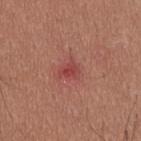Findings:
* image — ~15 mm crop, total-body skin-cancer survey
* patient — male, aged around 25
* site — the upper back
* TBP lesion metrics — a shape-asymmetry score of about 0.35 (0 = symmetric); a border-irregularity rating of about 3.5/10 and internal color variation of about 3 on a 0–10 scale; a nevus-likeness score of about 0/100 and a detector confidence of about 100 out of 100 that the crop contains a lesion
* lesion diameter — ~2.5 mm (longest diameter)